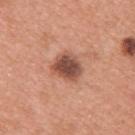Acquisition and patient details:
The tile uses white-light illumination. Located on the upper back. The subject is a male about 30 years old. An algorithmic analysis of the crop reported a lesion color around L≈50 a*≈24 b*≈28 in CIELAB. A 15 mm close-up extracted from a 3D total-body photography capture.